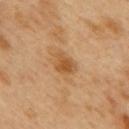| key | value |
|---|---|
| workup | total-body-photography surveillance lesion; no biopsy |
| lighting | cross-polarized illumination |
| subject | male, about 50 years old |
| body site | the mid back |
| imaging modality | total-body-photography crop, ~15 mm field of view |